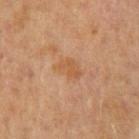Q: Was this lesion biopsied?
A: total-body-photography surveillance lesion; no biopsy
Q: What is the anatomic site?
A: the right upper arm
Q: What is the imaging modality?
A: total-body-photography crop, ~15 mm field of view
Q: Who is the patient?
A: male, aged 63–67